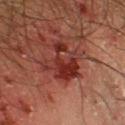This lesion was catalogued during total-body skin photography and was not selected for biopsy.
From the leg.
A 15 mm close-up tile from a total-body photography series done for melanoma screening.
Longest diameter approximately 4.5 mm.
Imaged with cross-polarized lighting.
The patient is a male roughly 65 years of age.